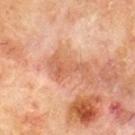Imaged during a routine full-body skin examination; the lesion was not biopsied and no histopathology is available. A 15 mm crop from a total-body photograph taken for skin-cancer surveillance. Located on the upper back. A male patient, roughly 70 years of age. The lesion's longest dimension is about 5 mm.